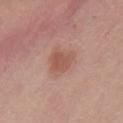• workup · total-body-photography surveillance lesion; no biopsy
• lesion size · ~3 mm (longest diameter)
• body site · the front of the torso
• subject · female, approximately 60 years of age
• acquisition · ~15 mm crop, total-body skin-cancer survey